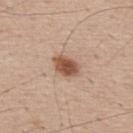No biopsy was performed on this lesion — it was imaged during a full skin examination and was not determined to be concerning. Automated tile analysis of the lesion measured a lesion area of about 6 mm², a shape eccentricity near 0.75, and a symmetry-axis asymmetry near 0.25. The software also gave about 15 CIELAB-L* units darker than the surrounding skin. The software also gave a border-irregularity index near 2/10, internal color variation of about 3.5 on a 0–10 scale, and a peripheral color-asymmetry measure near 1. The tile uses white-light illumination. About 3.5 mm across. A male patient, aged 53–57. Cropped from a total-body skin-imaging series; the visible field is about 15 mm.